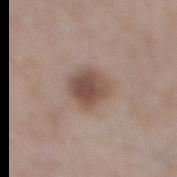Q: How was the tile lit?
A: white-light
Q: What kind of image is this?
A: ~15 mm tile from a whole-body skin photo
Q: What is the lesion's diameter?
A: about 4 mm
Q: Lesion location?
A: the leg
Q: Patient demographics?
A: female, aged approximately 60